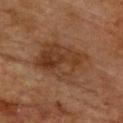Q: Is there a histopathology result?
A: no biopsy performed (imaged during a skin exam)
Q: Lesion location?
A: the chest
Q: Automated lesion metrics?
A: a footprint of about 26 mm², an outline eccentricity of about 0.7 (0 = round, 1 = elongated), and two-axis asymmetry of about 0.2
Q: Who is the patient?
A: male, in their mid- to late 70s
Q: Illumination type?
A: cross-polarized illumination
Q: How large is the lesion?
A: about 6.5 mm
Q: What kind of image is this?
A: total-body-photography crop, ~15 mm field of view